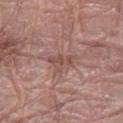biopsy status: total-body-photography surveillance lesion; no biopsy
image source: total-body-photography crop, ~15 mm field of view
subject: male, aged approximately 65
location: the left forearm